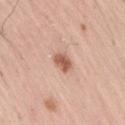This lesion was catalogued during total-body skin photography and was not selected for biopsy.
From the right thigh.
Cropped from a whole-body photographic skin survey; the tile spans about 15 mm.
The lesion's longest dimension is about 2.5 mm.
The subject is a male approximately 55 years of age.